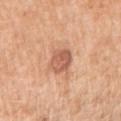Captured during whole-body skin photography for melanoma surveillance; the lesion was not biopsied. A 15 mm close-up tile from a total-body photography series done for melanoma screening. The lesion is on the right upper arm. A female subject about 55 years old. Captured under white-light illumination.